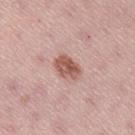Part of a total-body skin-imaging series; this lesion was reviewed on a skin check and was not flagged for biopsy. The lesion's longest dimension is about 3.5 mm. A female patient, aged 38 to 42. Imaged with white-light lighting. From the right thigh. An algorithmic analysis of the crop reported a shape eccentricity near 0.7 and two-axis asymmetry of about 0.2. And it measured an average lesion color of about L≈56 a*≈23 b*≈26 (CIELAB), roughly 13 lightness units darker than nearby skin, and a lesion-to-skin contrast of about 8.5 (normalized; higher = more distinct). A 15 mm close-up extracted from a 3D total-body photography capture.